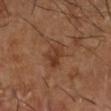Q: Was this lesion biopsied?
A: total-body-photography surveillance lesion; no biopsy
Q: Automated lesion metrics?
A: a peripheral color-asymmetry measure near 1.5; a classifier nevus-likeness of about 15/100 and a lesion-detection confidence of about 100/100
Q: What lighting was used for the tile?
A: cross-polarized illumination
Q: What kind of image is this?
A: ~15 mm crop, total-body skin-cancer survey
Q: Lesion size?
A: ≈3.5 mm
Q: Lesion location?
A: the right lower leg
Q: Patient demographics?
A: male, in their mid- to late 60s